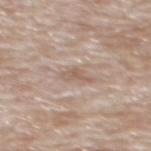* follow-up — catalogued during a skin exam; not biopsied
* size — ≈3 mm
* site — the back
* acquisition — ~15 mm tile from a whole-body skin photo
* patient — male, in their 80s